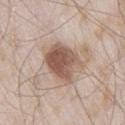  biopsy_status: not biopsied; imaged during a skin examination
  patient:
    sex: male
    age_approx: 45
  site: right thigh
  image:
    source: total-body photography crop
    field_of_view_mm: 15
  lesion_size:
    long_diameter_mm_approx: 5.5
  lighting: white-light